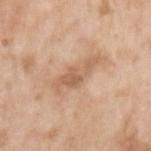Q: Was this lesion biopsied?
A: no biopsy performed (imaged during a skin exam)
Q: What is the anatomic site?
A: the left upper arm
Q: What kind of image is this?
A: 15 mm crop, total-body photography
Q: Patient demographics?
A: female, aged approximately 75